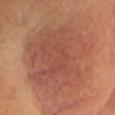biopsy status — total-body-photography surveillance lesion; no biopsy
anatomic site — the head or neck
diameter — about 11.5 mm
subject — female, approximately 60 years of age
image — ~15 mm crop, total-body skin-cancer survey
lighting — cross-polarized illumination